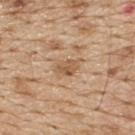Part of a total-body skin-imaging series; this lesion was reviewed on a skin check and was not flagged for biopsy.
A roughly 15 mm field-of-view crop from a total-body skin photograph.
The lesion-visualizer software estimated a shape eccentricity near 0.75 and two-axis asymmetry of about 0.25. The software also gave border irregularity of about 2.5 on a 0–10 scale, internal color variation of about 2.5 on a 0–10 scale, and radial color variation of about 1. The software also gave an automated nevus-likeness rating near 5 out of 100 and lesion-presence confidence of about 95/100.
The lesion is on the upper back.
Longest diameter approximately 3 mm.
A male subject roughly 70 years of age.
Captured under white-light illumination.